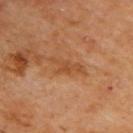Impression:
Part of a total-body skin-imaging series; this lesion was reviewed on a skin check and was not flagged for biopsy.
Context:
This image is a 15 mm lesion crop taken from a total-body photograph. The total-body-photography lesion software estimated a shape eccentricity near 0.9 and a symmetry-axis asymmetry near 0.55. The lesion is located on the upper back. Captured under cross-polarized illumination. A male subject, about 60 years old.